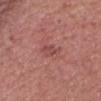The lesion was tiled from a total-body skin photograph and was not biopsied. This is a white-light tile. A 15 mm close-up tile from a total-body photography series done for melanoma screening. Located on the head or neck. Longest diameter approximately 3 mm. The total-body-photography lesion software estimated a lesion area of about 3.5 mm², an outline eccentricity of about 0.85 (0 = round, 1 = elongated), and two-axis asymmetry of about 0.25. The software also gave border irregularity of about 3 on a 0–10 scale and internal color variation of about 3 on a 0–10 scale. A male subject, aged approximately 70.